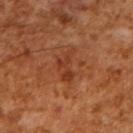Imaged with cross-polarized lighting. The subject is a male aged 63–67. A lesion tile, about 15 mm wide, cut from a 3D total-body photograph. The lesion's longest dimension is about 4 mm.